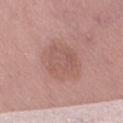{
  "biopsy_status": "not biopsied; imaged during a skin examination",
  "image": {
    "source": "total-body photography crop",
    "field_of_view_mm": 15
  },
  "patient": {
    "sex": "female",
    "age_approx": 60
  },
  "site": "leg"
}